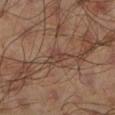Findings:
– size: about 3 mm
– image-analysis metrics: a lesion area of about 4 mm², an outline eccentricity of about 0.85 (0 = round, 1 = elongated), and two-axis asymmetry of about 0.4; a nevus-likeness score of about 0/100 and a lesion-detection confidence of about 60/100
– patient: male, in their mid- to late 40s
– image source: ~15 mm tile from a whole-body skin photo
– location: the leg
– illumination: cross-polarized illumination